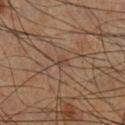Q: Is there a histopathology result?
A: total-body-photography surveillance lesion; no biopsy
Q: What did automated image analysis measure?
A: a lesion–skin lightness drop of about 7 and a normalized border contrast of about 5.5; a classifier nevus-likeness of about 0/100 and a detector confidence of about 10 out of 100 that the crop contains a lesion
Q: What lighting was used for the tile?
A: cross-polarized
Q: Who is the patient?
A: male, aged approximately 60
Q: How large is the lesion?
A: ~1.5 mm (longest diameter)
Q: What is the anatomic site?
A: the right lower leg
Q: What is the imaging modality?
A: ~15 mm tile from a whole-body skin photo